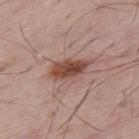Q: Is there a histopathology result?
A: no biopsy performed (imaged during a skin exam)
Q: Illumination type?
A: white-light
Q: What is the anatomic site?
A: the upper back
Q: What kind of image is this?
A: ~15 mm crop, total-body skin-cancer survey
Q: Who is the patient?
A: male, in their mid-60s
Q: What is the lesion's diameter?
A: ≈5 mm